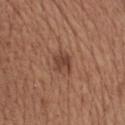Captured under white-light illumination. The lesion's longest dimension is about 2.5 mm. A lesion tile, about 15 mm wide, cut from a 3D total-body photograph. The lesion is on the chest. A male subject, about 65 years old. Automated image analysis of the tile measured a lesion area of about 5.5 mm² and a shape eccentricity near 0.55. And it measured border irregularity of about 1.5 on a 0–10 scale and internal color variation of about 3.5 on a 0–10 scale.